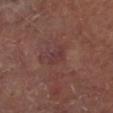Q: Is there a histopathology result?
A: imaged on a skin check; not biopsied
Q: How was the tile lit?
A: cross-polarized illumination
Q: Automated lesion metrics?
A: a shape-asymmetry score of about 0.25 (0 = symmetric)
Q: Who is the patient?
A: male, aged approximately 65
Q: Lesion location?
A: the leg
Q: How large is the lesion?
A: about 3 mm
Q: What kind of image is this?
A: ~15 mm crop, total-body skin-cancer survey